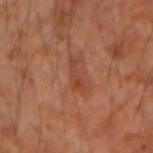Clinical impression: Captured during whole-body skin photography for melanoma surveillance; the lesion was not biopsied. Context: From the left forearm. The lesion-visualizer software estimated roughly 7 lightness units darker than nearby skin and a normalized lesion–skin contrast near 6. Measured at roughly 3.5 mm in maximum diameter. This image is a 15 mm lesion crop taken from a total-body photograph. A male patient, aged 53 to 57. Captured under cross-polarized illumination.